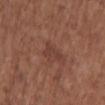Q: Was this lesion biopsied?
A: imaged on a skin check; not biopsied
Q: What are the patient's age and sex?
A: female, aged 73–77
Q: Where on the body is the lesion?
A: the upper back
Q: What is the imaging modality?
A: ~15 mm tile from a whole-body skin photo
Q: Automated lesion metrics?
A: a mean CIELAB color near L≈39 a*≈22 b*≈25, a lesion–skin lightness drop of about 6, and a normalized lesion–skin contrast near 5.5; a classifier nevus-likeness of about 0/100 and a lesion-detection confidence of about 100/100
Q: Lesion size?
A: ≈2.5 mm
Q: Illumination type?
A: white-light illumination